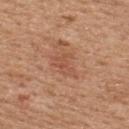Imaged during a routine full-body skin examination; the lesion was not biopsied and no histopathology is available. Imaged with white-light lighting. A female patient, approximately 45 years of age. The total-body-photography lesion software estimated an area of roughly 5.5 mm², a shape eccentricity near 0.8, and a shape-asymmetry score of about 0.4 (0 = symmetric). It also reported an automated nevus-likeness rating near 0 out of 100 and a lesion-detection confidence of about 100/100. From the upper back. Cropped from a whole-body photographic skin survey; the tile spans about 15 mm.